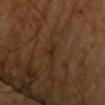Clinical impression: Part of a total-body skin-imaging series; this lesion was reviewed on a skin check and was not flagged for biopsy. Context: A lesion tile, about 15 mm wide, cut from a 3D total-body photograph. A female subject aged 58–62. The lesion is located on the chest.